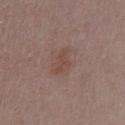Recorded during total-body skin imaging; not selected for excision or biopsy. Imaged with white-light lighting. The total-body-photography lesion software estimated a lesion color around L≈46 a*≈18 b*≈24 in CIELAB, about 6 CIELAB-L* units darker than the surrounding skin, and a lesion-to-skin contrast of about 6 (normalized; higher = more distinct). The software also gave a within-lesion color-variation index near 0.5/10 and peripheral color asymmetry of about 0.5. It also reported an automated nevus-likeness rating near 0 out of 100 and a detector confidence of about 100 out of 100 that the crop contains a lesion. The subject is a female aged around 50. A close-up tile cropped from a whole-body skin photograph, about 15 mm across. About 3.5 mm across. From the chest.